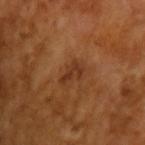<tbp_lesion>
<lesion_size>
  <long_diameter_mm_approx>3.0</long_diameter_mm_approx>
</lesion_size>
<automated_metrics>
  <area_mm2_approx>4.0</area_mm2_approx>
  <border_irregularity_0_10>5.0</border_irregularity_0_10>
  <color_variation_0_10>1.5</color_variation_0_10>
  <peripheral_color_asymmetry>0.5</peripheral_color_asymmetry>
  <nevus_likeness_0_100>0</nevus_likeness_0_100>
  <lesion_detection_confidence_0_100>100</lesion_detection_confidence_0_100>
</automated_metrics>
<lighting>cross-polarized</lighting>
<image>
  <source>total-body photography crop</source>
  <field_of_view_mm>15</field_of_view_mm>
</image>
<patient>
  <sex>male</sex>
  <age_approx>65</age_approx>
</patient>
</tbp_lesion>